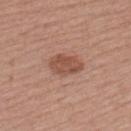Image and clinical context:
A female patient, roughly 55 years of age. A lesion tile, about 15 mm wide, cut from a 3D total-body photograph. The recorded lesion diameter is about 4 mm. An algorithmic analysis of the crop reported a lesion color around L≈51 a*≈23 b*≈28 in CIELAB, roughly 10 lightness units darker than nearby skin, and a lesion-to-skin contrast of about 7.5 (normalized; higher = more distinct). And it measured a classifier nevus-likeness of about 60/100 and a detector confidence of about 100 out of 100 that the crop contains a lesion. The tile uses white-light illumination. On the left upper arm.